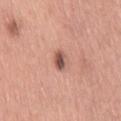This lesion was catalogued during total-body skin photography and was not selected for biopsy.
Automated image analysis of the tile measured a footprint of about 4 mm² and a shape eccentricity near 0.8.
Imaged with white-light lighting.
From the right thigh.
The patient is a female approximately 55 years of age.
A 15 mm crop from a total-body photograph taken for skin-cancer surveillance.
About 3 mm across.